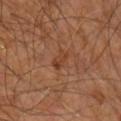Clinical impression: Imaged during a routine full-body skin examination; the lesion was not biopsied and no histopathology is available. Context: A roughly 15 mm field-of-view crop from a total-body skin photograph. Measured at roughly 2.5 mm in maximum diameter. On the right leg. The tile uses cross-polarized illumination. A male patient about 60 years old. Automated image analysis of the tile measured an average lesion color of about L≈39 a*≈22 b*≈31 (CIELAB). And it measured a nevus-likeness score of about 0/100 and a detector confidence of about 100 out of 100 that the crop contains a lesion.